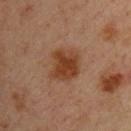Q: Was this lesion biopsied?
A: imaged on a skin check; not biopsied
Q: What is the imaging modality?
A: ~15 mm crop, total-body skin-cancer survey
Q: What is the lesion's diameter?
A: ~4 mm (longest diameter)
Q: Where on the body is the lesion?
A: the upper back
Q: Who is the patient?
A: male, aged around 35
Q: Automated lesion metrics?
A: a footprint of about 12 mm², a shape eccentricity near 0.6, and two-axis asymmetry of about 0.25; internal color variation of about 3.5 on a 0–10 scale and a peripheral color-asymmetry measure near 1; a classifier nevus-likeness of about 95/100 and a lesion-detection confidence of about 100/100